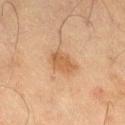Q: Was this lesion biopsied?
A: total-body-photography surveillance lesion; no biopsy
Q: How was the tile lit?
A: cross-polarized
Q: What kind of image is this?
A: 15 mm crop, total-body photography
Q: Patient demographics?
A: male, aged 68–72
Q: Lesion location?
A: the right thigh